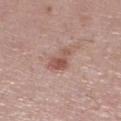| feature | finding |
|---|---|
| workup | catalogued during a skin exam; not biopsied |
| site | the right lower leg |
| lesion diameter | ≈3 mm |
| acquisition | 15 mm crop, total-body photography |
| automated metrics | an automated nevus-likeness rating near 65 out of 100 and lesion-presence confidence of about 100/100 |
| subject | female, in their mid-60s |